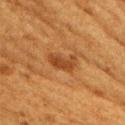biopsy status — catalogued during a skin exam; not biopsied | subject — female, about 50 years old | site — the arm | automated metrics — a lesion area of about 5 mm², a shape eccentricity near 0.9, and a symmetry-axis asymmetry near 0.3; roughly 9 lightness units darker than nearby skin and a normalized lesion–skin contrast near 8; a lesion-detection confidence of about 100/100 | lesion diameter — about 3.5 mm | illumination — cross-polarized illumination | acquisition — ~15 mm tile from a whole-body skin photo.